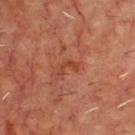Imaged during a routine full-body skin examination; the lesion was not biopsied and no histopathology is available. A male patient, in their mid- to late 60s. The lesion is on the upper back. A lesion tile, about 15 mm wide, cut from a 3D total-body photograph.